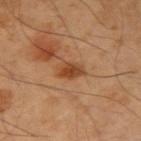  biopsy_status: not biopsied; imaged during a skin examination
  image:
    source: total-body photography crop
    field_of_view_mm: 15
  lesion_size:
    long_diameter_mm_approx: 3.0
  patient:
    sex: male
    age_approx: 50
  site: left arm
  lighting: cross-polarized